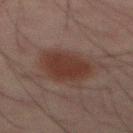| feature | finding |
|---|---|
| follow-up | imaged on a skin check; not biopsied |
| size | about 5 mm |
| subject | male, approximately 65 years of age |
| lighting | cross-polarized |
| acquisition | total-body-photography crop, ~15 mm field of view |
| automated lesion analysis | an automated nevus-likeness rating near 95 out of 100 |
| location | the mid back |